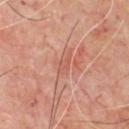Imaged during a routine full-body skin examination; the lesion was not biopsied and no histopathology is available. A close-up tile cropped from a whole-body skin photograph, about 15 mm across. This is a cross-polarized tile. The subject is a male approximately 65 years of age. From the chest.